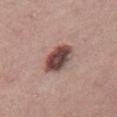| key | value |
|---|---|
| notes | no biopsy performed (imaged during a skin exam) |
| image | ~15 mm crop, total-body skin-cancer survey |
| lesion diameter | about 4.5 mm |
| tile lighting | white-light illumination |
| anatomic site | the chest |
| patient | male, aged 38 to 42 |